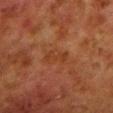biopsy status = catalogued during a skin exam; not biopsied | tile lighting = cross-polarized illumination | image = total-body-photography crop, ~15 mm field of view | size = about 3.5 mm | anatomic site = the left lower leg | patient = male, about 80 years old.